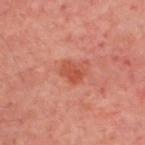Findings:
– follow-up — total-body-photography surveillance lesion; no biopsy
– patient — in their mid- to late 50s
– automated metrics — border irregularity of about 2.5 on a 0–10 scale and a color-variation rating of about 2.5/10
– body site — the upper back
– tile lighting — cross-polarized illumination
– imaging modality — ~15 mm crop, total-body skin-cancer survey